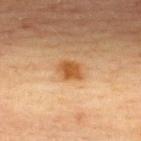Case summary:
– follow-up — no biopsy performed (imaged during a skin exam)
– imaging modality — ~15 mm tile from a whole-body skin photo
– lighting — cross-polarized
– patient — female, aged 63–67
– lesion diameter — about 3 mm
– anatomic site — the upper back
– TBP lesion metrics — a mean CIELAB color near L≈47 a*≈21 b*≈37 and a normalized lesion–skin contrast near 8.5; a border-irregularity index near 2.5/10 and peripheral color asymmetry of about 0.5; a classifier nevus-likeness of about 95/100 and lesion-presence confidence of about 100/100